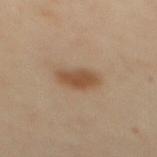| key | value |
|---|---|
| workup | total-body-photography surveillance lesion; no biopsy |
| patient | female, in their 40s |
| image-analysis metrics | a lesion color around L≈51 a*≈18 b*≈33 in CIELAB, a lesion–skin lightness drop of about 11, and a normalized lesion–skin contrast near 8.5; border irregularity of about 2 on a 0–10 scale, a color-variation rating of about 2.5/10, and radial color variation of about 1 |
| body site | the back |
| imaging modality | total-body-photography crop, ~15 mm field of view |